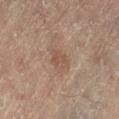Imaged during a routine full-body skin examination; the lesion was not biopsied and no histopathology is available.
An algorithmic analysis of the crop reported an area of roughly 4.5 mm², an eccentricity of roughly 0.35, and a shape-asymmetry score of about 0.25 (0 = symmetric). It also reported a border-irregularity index near 2.5/10, a within-lesion color-variation index near 2.5/10, and peripheral color asymmetry of about 1. The analysis additionally found an automated nevus-likeness rating near 0 out of 100 and lesion-presence confidence of about 100/100.
A female patient roughly 80 years of age.
Cropped from a whole-body photographic skin survey; the tile spans about 15 mm.
On the back.
About 2.5 mm across.